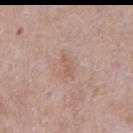| field | value |
|---|---|
| follow-up | total-body-photography surveillance lesion; no biopsy |
| tile lighting | white-light |
| site | the abdomen |
| lesion size | ≈2.5 mm |
| automated lesion analysis | a lesion–skin lightness drop of about 7 and a lesion-to-skin contrast of about 5 (normalized; higher = more distinct) |
| subject | male, aged 58–62 |
| image | ~15 mm crop, total-body skin-cancer survey |